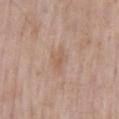biopsy_status: not biopsied; imaged during a skin examination